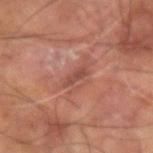  biopsy_status: not biopsied; imaged during a skin examination
  patient:
    sex: male
    age_approx: 60
  lesion_size:
    long_diameter_mm_approx: 3.0
  automated_metrics:
    cielab_L: 45
    cielab_a: 24
    cielab_b: 26
    vs_skin_darker_L: 8.0
    vs_skin_contrast_norm: 6.5
    border_irregularity_0_10: 3.5
    color_variation_0_10: 0.0
    peripheral_color_asymmetry: 0.0
    nevus_likeness_0_100: 0
    lesion_detection_confidence_0_100: 90
  image:
    source: total-body photography crop
    field_of_view_mm: 15
  lighting: cross-polarized
  site: right arm